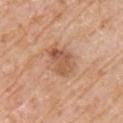follow-up = imaged on a skin check; not biopsied
automated lesion analysis = a border-irregularity index near 3/10, a color-variation rating of about 3.5/10, and a peripheral color-asymmetry measure near 1
imaging modality = ~15 mm tile from a whole-body skin photo
patient = male, in their mid- to late 70s
body site = the right upper arm
size = about 4 mm
illumination = white-light illumination